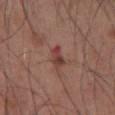No biopsy was performed on this lesion — it was imaged during a full skin examination and was not determined to be concerning.
This image is a 15 mm lesion crop taken from a total-body photograph.
A male subject in their mid-50s.
Captured under white-light illumination.
Located on the abdomen.
Measured at roughly 3 mm in maximum diameter.